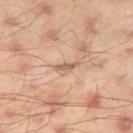{"biopsy_status": "not biopsied; imaged during a skin examination", "site": "right thigh", "automated_metrics": {"border_irregularity_0_10": 4.0, "color_variation_0_10": 0.5, "peripheral_color_asymmetry": 0.0, "nevus_likeness_0_100": 0}, "image": {"source": "total-body photography crop", "field_of_view_mm": 15}, "patient": {"sex": "male", "age_approx": 45}, "lighting": "cross-polarized"}